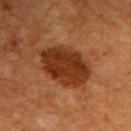Imaged during a routine full-body skin examination; the lesion was not biopsied and no histopathology is available.
A 15 mm close-up extracted from a 3D total-body photography capture.
An algorithmic analysis of the crop reported a footprint of about 21 mm², an outline eccentricity of about 0.75 (0 = round, 1 = elongated), and a shape-asymmetry score of about 0.15 (0 = symmetric). The analysis additionally found roughly 11 lightness units darker than nearby skin and a normalized border contrast of about 11. And it measured a border-irregularity rating of about 1.5/10 and a within-lesion color-variation index near 3/10.
Located on the upper back.
A female subject, aged 53 to 57.
The lesion's longest dimension is about 6.5 mm.
The tile uses cross-polarized illumination.